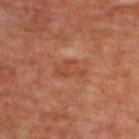TBP lesion metrics = a classifier nevus-likeness of about 0/100 and lesion-presence confidence of about 100/100
subject = female, aged around 45
body site = the upper back
acquisition = 15 mm crop, total-body photography